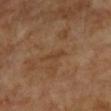Recorded during total-body skin imaging; not selected for excision or biopsy. Imaged with cross-polarized lighting. Approximately 3 mm at its widest. A 15 mm crop from a total-body photograph taken for skin-cancer surveillance. A female patient, in their 70s. The lesion is located on the left forearm.